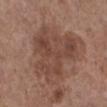follow-up — catalogued during a skin exam; not biopsied
site — the leg
diameter — ≈8 mm
automated metrics — an area of roughly 33 mm², a shape eccentricity near 0.45, and a shape-asymmetry score of about 0.5 (0 = symmetric); a color-variation rating of about 4.5/10 and peripheral color asymmetry of about 1.5
lighting — white-light illumination
acquisition — ~15 mm tile from a whole-body skin photo
patient — female, in their mid-60s